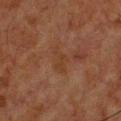biopsy status: no biopsy performed (imaged during a skin exam); subject: male, aged around 60; location: the chest; image: ~15 mm crop, total-body skin-cancer survey.